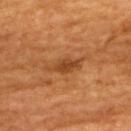| field | value |
|---|---|
| workup | catalogued during a skin exam; not biopsied |
| TBP lesion metrics | an area of roughly 6 mm² and an outline eccentricity of about 0.9 (0 = round, 1 = elongated); a lesion color around L≈41 a*≈23 b*≈37 in CIELAB, about 9 CIELAB-L* units darker than the surrounding skin, and a normalized lesion–skin contrast near 7.5 |
| image source | ~15 mm tile from a whole-body skin photo |
| subject | male, aged around 60 |
| illumination | cross-polarized |
| body site | the back |